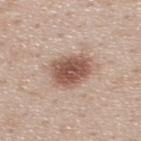workup: total-body-photography surveillance lesion; no biopsy
subject: male, roughly 40 years of age
body site: the upper back
imaging modality: ~15 mm tile from a whole-body skin photo